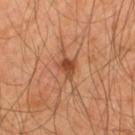Cropped from a whole-body photographic skin survey; the tile spans about 15 mm.
On the mid back.
Measured at roughly 2.5 mm in maximum diameter.
A male patient, aged 43 to 47.